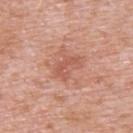Impression: The lesion was tiled from a total-body skin photograph and was not biopsied. Clinical summary: A male patient, approximately 50 years of age. The lesion is located on the upper back. An algorithmic analysis of the crop reported an average lesion color of about L≈56 a*≈27 b*≈30 (CIELAB) and a lesion-to-skin contrast of about 5.5 (normalized; higher = more distinct). And it measured an automated nevus-likeness rating near 0 out of 100 and lesion-presence confidence of about 100/100. Imaged with white-light lighting. The recorded lesion diameter is about 3.5 mm. A 15 mm close-up tile from a total-body photography series done for melanoma screening.